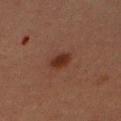  biopsy_status: not biopsied; imaged during a skin examination
  image:
    source: total-body photography crop
    field_of_view_mm: 15
  patient:
    sex: female
    age_approx: 55
  site: right upper arm
  lighting: cross-polarized